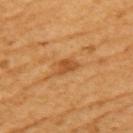Findings:
- follow-up · imaged on a skin check; not biopsied
- body site · the left upper arm
- patient · female, aged 53–57
- image source · 15 mm crop, total-body photography
- lighting · cross-polarized
- TBP lesion metrics · an area of roughly 4 mm², a shape eccentricity near 0.8, and a symmetry-axis asymmetry near 0.35; a lesion color around L≈53 a*≈26 b*≈45 in CIELAB and a lesion–skin lightness drop of about 10; a border-irregularity rating of about 3/10, a color-variation rating of about 1.5/10, and radial color variation of about 0.5
- size · ~3 mm (longest diameter)